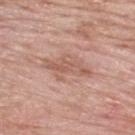– biopsy status: imaged on a skin check; not biopsied
– subject: male, in their 60s
– lighting: white-light illumination
– site: the upper back
– automated lesion analysis: a lesion area of about 11 mm², an outline eccentricity of about 0.85 (0 = round, 1 = elongated), and a symmetry-axis asymmetry near 0.3; an average lesion color of about L≈58 a*≈21 b*≈27 (CIELAB), roughly 8 lightness units darker than nearby skin, and a normalized border contrast of about 5.5; border irregularity of about 4.5 on a 0–10 scale and a within-lesion color-variation index near 3.5/10
– acquisition: ~15 mm tile from a whole-body skin photo
– lesion diameter: ~6 mm (longest diameter)